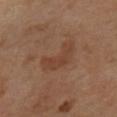Assessment: Imaged during a routine full-body skin examination; the lesion was not biopsied and no histopathology is available. Background: Imaged with cross-polarized lighting. From the left lower leg. A close-up tile cropped from a whole-body skin photograph, about 15 mm across. About 4 mm across. A female subject aged 48–52.